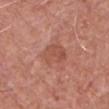biopsy status: no biopsy performed (imaged during a skin exam)
imaging modality: ~15 mm tile from a whole-body skin photo
TBP lesion metrics: an area of roughly 7 mm², an outline eccentricity of about 0.65 (0 = round, 1 = elongated), and a shape-asymmetry score of about 0.2 (0 = symmetric); an average lesion color of about L≈52 a*≈25 b*≈30 (CIELAB)
patient: male, aged around 80
location: the chest
lesion diameter: about 3.5 mm